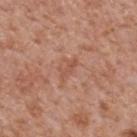This is a white-light tile.
This image is a 15 mm lesion crop taken from a total-body photograph.
The lesion is located on the back.
An algorithmic analysis of the crop reported a border-irregularity index near 5/10, internal color variation of about 0 on a 0–10 scale, and a peripheral color-asymmetry measure near 0.
Approximately 3 mm at its widest.
A male subject aged around 65.